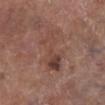Part of a total-body skin-imaging series; this lesion was reviewed on a skin check and was not flagged for biopsy.
A male patient, aged approximately 70.
Measured at roughly 6.5 mm in maximum diameter.
This image is a 15 mm lesion crop taken from a total-body photograph.
The tile uses white-light illumination.
Located on the left lower leg.